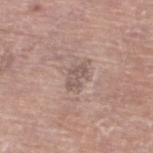The lesion was tiled from a total-body skin photograph and was not biopsied.
The lesion-visualizer software estimated a normalized border contrast of about 6. And it measured border irregularity of about 3.5 on a 0–10 scale and a within-lesion color-variation index near 2.5/10. It also reported an automated nevus-likeness rating near 0 out of 100 and a lesion-detection confidence of about 95/100.
Measured at roughly 3 mm in maximum diameter.
The lesion is on the left lower leg.
Cropped from a whole-body photographic skin survey; the tile spans about 15 mm.
A male subject in their mid- to late 70s.